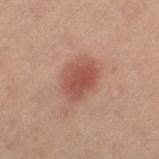Findings:
– workup: no biopsy performed (imaged during a skin exam)
– lesion size: ≈4.5 mm
– anatomic site: the leg
– lighting: cross-polarized
– acquisition: total-body-photography crop, ~15 mm field of view
– subject: female, about 40 years old
– TBP lesion metrics: an area of roughly 12 mm², an outline eccentricity of about 0.65 (0 = round, 1 = elongated), and two-axis asymmetry of about 0.2; a lesion color around L≈40 a*≈20 b*≈23 in CIELAB and about 9 CIELAB-L* units darker than the surrounding skin